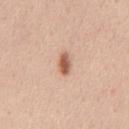Located on the chest. About 2.5 mm across. A male subject, about 45 years old. A lesion tile, about 15 mm wide, cut from a 3D total-body photograph. The tile uses white-light illumination.